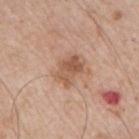follow-up=imaged on a skin check; not biopsied
lesion size=≈3.5 mm
anatomic site=the right upper arm
lighting=white-light illumination
image source=15 mm crop, total-body photography
patient=male, aged approximately 65
automated lesion analysis=an average lesion color of about L≈55 a*≈21 b*≈31 (CIELAB) and a lesion–skin lightness drop of about 10